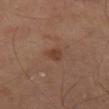Imaged during a routine full-body skin examination; the lesion was not biopsied and no histopathology is available.
Imaged with cross-polarized lighting.
The subject is a male roughly 70 years of age.
Automated image analysis of the tile measured an area of roughly 3.5 mm², a shape eccentricity near 0.55, and a symmetry-axis asymmetry near 0.3. The software also gave a border-irregularity index near 3/10 and a within-lesion color-variation index near 1/10.
A region of skin cropped from a whole-body photographic capture, roughly 15 mm wide.
About 2 mm across.
Located on the right thigh.